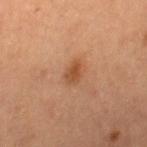Q: Is there a histopathology result?
A: catalogued during a skin exam; not biopsied
Q: Illumination type?
A: cross-polarized
Q: Where on the body is the lesion?
A: the right thigh
Q: What is the lesion's diameter?
A: ~2.5 mm (longest diameter)
Q: How was this image acquired?
A: ~15 mm tile from a whole-body skin photo
Q: What are the patient's age and sex?
A: female, aged 58 to 62